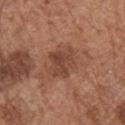Clinical summary:
The lesion is on the right upper arm. The patient is a male approximately 75 years of age. About 3.5 mm across. A region of skin cropped from a whole-body photographic capture, roughly 15 mm wide. This is a white-light tile. An algorithmic analysis of the crop reported a lesion area of about 8.5 mm² and a symmetry-axis asymmetry near 0.35. It also reported a classifier nevus-likeness of about 0/100.